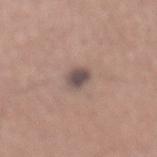A male subject roughly 45 years of age. A 15 mm crop from a total-body photograph taken for skin-cancer surveillance. About 2.5 mm across. The lesion is on the mid back. This is a white-light tile. The total-body-photography lesion software estimated a color-variation rating of about 2.5/10 and a peripheral color-asymmetry measure near 0.5.